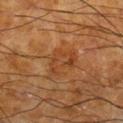| key | value |
|---|---|
| follow-up | no biopsy performed (imaged during a skin exam) |
| automated lesion analysis | an area of roughly 7 mm², a shape eccentricity near 0.7, and two-axis asymmetry of about 0.45; an average lesion color of about L≈34 a*≈20 b*≈31 (CIELAB) and a lesion–skin lightness drop of about 5; border irregularity of about 4.5 on a 0–10 scale, a color-variation rating of about 4/10, and peripheral color asymmetry of about 1.5 |
| size | ≈4 mm |
| location | the right lower leg |
| patient | male, aged approximately 65 |
| acquisition | 15 mm crop, total-body photography |
| illumination | cross-polarized illumination |